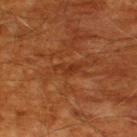Captured during whole-body skin photography for melanoma surveillance; the lesion was not biopsied. Imaged with cross-polarized lighting. Located on the upper back. Measured at roughly 3 mm in maximum diameter. A 15 mm close-up tile from a total-body photography series done for melanoma screening. The patient is a male aged around 60.